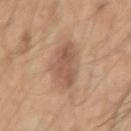Notes:
- follow-up — catalogued during a skin exam; not biopsied
- patient — male, aged 48 to 52
- lighting — white-light
- lesion size — about 4.5 mm
- site — the right upper arm
- imaging modality — ~15 mm tile from a whole-body skin photo